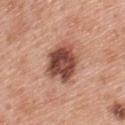notes = catalogued during a skin exam; not biopsied | lesion size = about 5 mm | acquisition = 15 mm crop, total-body photography | patient = male, approximately 60 years of age | location = the upper back | automated metrics = a lesion area of about 16 mm², an eccentricity of roughly 0.45, and a symmetry-axis asymmetry near 0.25; an average lesion color of about L≈48 a*≈24 b*≈28 (CIELAB), about 17 CIELAB-L* units darker than the surrounding skin, and a normalized border contrast of about 11.5; a border-irregularity rating of about 2.5/10.